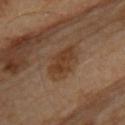No biopsy was performed on this lesion — it was imaged during a full skin examination and was not determined to be concerning. The total-body-photography lesion software estimated border irregularity of about 2.5 on a 0–10 scale, internal color variation of about 3 on a 0–10 scale, and radial color variation of about 1. Longest diameter approximately 5 mm. A male patient, in their mid- to late 80s or older. From the upper back. A 15 mm crop from a total-body photograph taken for skin-cancer surveillance.